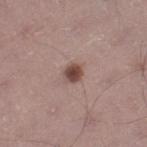Q: Is there a histopathology result?
A: catalogued during a skin exam; not biopsied
Q: Who is the patient?
A: male, in their mid-40s
Q: Lesion size?
A: ≈2.5 mm
Q: What is the imaging modality?
A: total-body-photography crop, ~15 mm field of view
Q: Where on the body is the lesion?
A: the left thigh